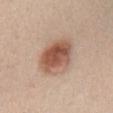Captured during whole-body skin photography for melanoma surveillance; the lesion was not biopsied. A female patient, in their mid-40s. From the chest. A 15 mm close-up extracted from a 3D total-body photography capture. The tile uses white-light illumination.